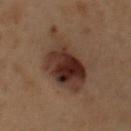Captured during whole-body skin photography for melanoma surveillance; the lesion was not biopsied.
A male subject, about 50 years old.
The lesion is on the abdomen.
Cropped from a whole-body photographic skin survey; the tile spans about 15 mm.